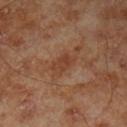biopsy_status: not biopsied; imaged during a skin examination
lighting: cross-polarized
lesion_size:
  long_diameter_mm_approx: 3.0
site: left lower leg
patient:
  sex: male
  age_approx: 70
image:
  source: total-body photography crop
  field_of_view_mm: 15